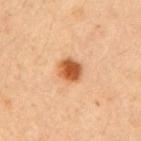notes: total-body-photography surveillance lesion; no biopsy | illumination: cross-polarized illumination | size: about 3 mm | acquisition: 15 mm crop, total-body photography | anatomic site: the arm | patient: female, aged 38–42.